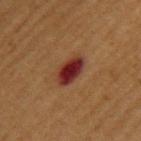Q: Was this lesion biopsied?
A: no biopsy performed (imaged during a skin exam)
Q: Lesion location?
A: the upper back
Q: How was this image acquired?
A: ~15 mm tile from a whole-body skin photo
Q: Who is the patient?
A: male, aged approximately 55